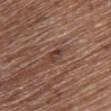Notes:
– biopsy status · total-body-photography surveillance lesion; no biopsy
– size · about 3 mm
– location · the chest
– lighting · white-light
– subject · female, aged 78 to 82
– automated lesion analysis · an average lesion color of about L≈39 a*≈20 b*≈25 (CIELAB), a lesion–skin lightness drop of about 9, and a lesion-to-skin contrast of about 7.5 (normalized; higher = more distinct); a nevus-likeness score of about 0/100 and a detector confidence of about 55 out of 100 that the crop contains a lesion
– acquisition · ~15 mm tile from a whole-body skin photo